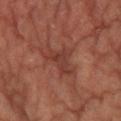The lesion was photographed on a routine skin check and not biopsied; there is no pathology result.
Measured at roughly 4.5 mm in maximum diameter.
A 15 mm close-up tile from a total-body photography series done for melanoma screening.
On the right lower leg.
The lesion-visualizer software estimated an outline eccentricity of about 0.8 (0 = round, 1 = elongated) and a shape-asymmetry score of about 0.6 (0 = symmetric). And it measured about 7 CIELAB-L* units darker than the surrounding skin and a lesion-to-skin contrast of about 6 (normalized; higher = more distinct). It also reported an automated nevus-likeness rating near 0 out of 100 and a lesion-detection confidence of about 90/100.
The subject is a female approximately 60 years of age.